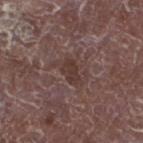Case summary:
- notes — imaged on a skin check; not biopsied